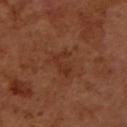Recorded during total-body skin imaging; not selected for excision or biopsy.